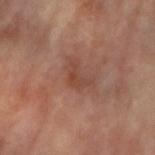Q: Was a biopsy performed?
A: catalogued during a skin exam; not biopsied
Q: Lesion size?
A: about 3 mm
Q: Who is the patient?
A: female, roughly 65 years of age
Q: How was the tile lit?
A: cross-polarized illumination
Q: Automated lesion metrics?
A: a shape-asymmetry score of about 0.6 (0 = symmetric); a lesion color around L≈45 a*≈23 b*≈29 in CIELAB, a lesion–skin lightness drop of about 7, and a normalized border contrast of about 5.5; border irregularity of about 6 on a 0–10 scale, a within-lesion color-variation index near 1/10, and peripheral color asymmetry of about 0.5; a lesion-detection confidence of about 100/100
Q: What is the imaging modality?
A: total-body-photography crop, ~15 mm field of view
Q: Where on the body is the lesion?
A: the right forearm